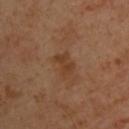Clinical impression: The lesion was photographed on a routine skin check and not biopsied; there is no pathology result. Context: A close-up tile cropped from a whole-body skin photograph, about 15 mm across. A male patient aged 43–47. Longest diameter approximately 3 mm. Captured under cross-polarized illumination. Located on the upper back.